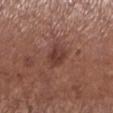The lesion was photographed on a routine skin check and not biopsied; there is no pathology result.
The tile uses white-light illumination.
The patient is a female approximately 55 years of age.
Located on the left lower leg.
A 15 mm crop from a total-body photograph taken for skin-cancer surveillance.
About 3 mm across.